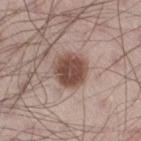Captured during whole-body skin photography for melanoma surveillance; the lesion was not biopsied.
Captured under white-light illumination.
A male patient, aged around 30.
On the right thigh.
The lesion's longest dimension is about 4 mm.
A 15 mm close-up extracted from a 3D total-body photography capture.